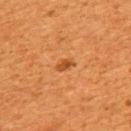Captured during whole-body skin photography for melanoma surveillance; the lesion was not biopsied. On the upper back. The subject is a male aged approximately 45. A region of skin cropped from a whole-body photographic capture, roughly 15 mm wide.